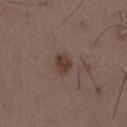follow-up: catalogued during a skin exam; not biopsied
lighting: white-light
image-analysis metrics: a border-irregularity rating of about 2/10 and a within-lesion color-variation index near 2.5/10; a nevus-likeness score of about 90/100 and lesion-presence confidence of about 100/100
site: the back
lesion size: ~3 mm (longest diameter)
acquisition: 15 mm crop, total-body photography
subject: male, approximately 50 years of age